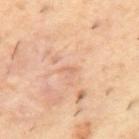Clinical impression:
Captured during whole-body skin photography for melanoma surveillance; the lesion was not biopsied.
Clinical summary:
Automated image analysis of the tile measured a footprint of about 1 mm² and a shape eccentricity near 0.9. It also reported internal color variation of about 0 on a 0–10 scale and a peripheral color-asymmetry measure near 0. The software also gave a nevus-likeness score of about 0/100 and a detector confidence of about 100 out of 100 that the crop contains a lesion. The lesion is on the back. Measured at roughly 1.5 mm in maximum diameter. A male patient, roughly 55 years of age. Cropped from a whole-body photographic skin survey; the tile spans about 15 mm.